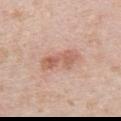Captured during whole-body skin photography for melanoma surveillance; the lesion was not biopsied.
This is a white-light tile.
A male subject, aged 38–42.
The lesion is located on the chest.
A 15 mm close-up extracted from a 3D total-body photography capture.